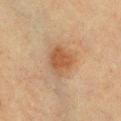This lesion was catalogued during total-body skin photography and was not selected for biopsy. This is a cross-polarized tile. Cropped from a total-body skin-imaging series; the visible field is about 15 mm. A female patient aged approximately 55. On the chest. The lesion-visualizer software estimated a lesion area of about 7 mm² and two-axis asymmetry of about 0.25. And it measured a lesion color around L≈42 a*≈19 b*≈30 in CIELAB and a normalized border contrast of about 7.5. The analysis additionally found border irregularity of about 3 on a 0–10 scale, internal color variation of about 1.5 on a 0–10 scale, and peripheral color asymmetry of about 0.5. The software also gave an automated nevus-likeness rating near 90 out of 100.